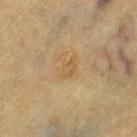The lesion was photographed on a routine skin check and not biopsied; there is no pathology result.
The lesion is on the leg.
Longest diameter approximately 2.5 mm.
A female subject about 55 years old.
A 15 mm close-up tile from a total-body photography series done for melanoma screening.